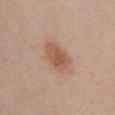Q: Is there a histopathology result?
A: total-body-photography surveillance lesion; no biopsy
Q: What is the anatomic site?
A: the chest
Q: Illumination type?
A: white-light
Q: Patient demographics?
A: female, about 25 years old
Q: What kind of image is this?
A: ~15 mm crop, total-body skin-cancer survey
Q: How large is the lesion?
A: ~4.5 mm (longest diameter)